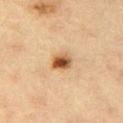workup: total-body-photography surveillance lesion; no biopsy | lighting: cross-polarized | location: the leg | automated lesion analysis: a footprint of about 5.5 mm² and a shape-asymmetry score of about 0.2 (0 = symmetric); a mean CIELAB color near L≈48 a*≈18 b*≈34, roughly 14 lightness units darker than nearby skin, and a lesion-to-skin contrast of about 10.5 (normalized; higher = more distinct); a classifier nevus-likeness of about 100/100 | image: ~15 mm tile from a whole-body skin photo | subject: female, about 65 years old | lesion size: ~3 mm (longest diameter).